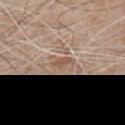This is a white-light tile. From the chest. Automated image analysis of the tile measured border irregularity of about 4 on a 0–10 scale, a within-lesion color-variation index near 1/10, and a peripheral color-asymmetry measure near 0.5. And it measured a nevus-likeness score of about 0/100 and a lesion-detection confidence of about 70/100. A male patient in their 80s. Approximately 2.5 mm at its widest. A roughly 15 mm field-of-view crop from a total-body skin photograph.